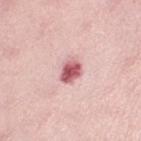The lesion was tiled from a total-body skin photograph and was not biopsied. Imaged with white-light lighting. A female patient, aged 28 to 32. Longest diameter approximately 3 mm. A 15 mm close-up tile from a total-body photography series done for melanoma screening. The lesion is located on the left thigh.